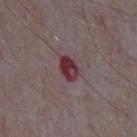Acquisition and patient details: A lesion tile, about 15 mm wide, cut from a 3D total-body photograph. Located on the abdomen. The lesion-visualizer software estimated a mean CIELAB color near L≈34 a*≈26 b*≈14, a lesion–skin lightness drop of about 13, and a normalized border contrast of about 11. The analysis additionally found a color-variation rating of about 7/10 and a peripheral color-asymmetry measure near 2.5. The patient is a male about 65 years old. The tile uses white-light illumination. The recorded lesion diameter is about 3 mm.